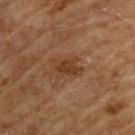Imaged with cross-polarized lighting.
The lesion is located on the back.
A 15 mm crop from a total-body photograph taken for skin-cancer surveillance.
The patient is a male aged around 65.
Automated image analysis of the tile measured a mean CIELAB color near L≈33 a*≈19 b*≈29, a lesion–skin lightness drop of about 7, and a normalized lesion–skin contrast near 7.5. The analysis additionally found an automated nevus-likeness rating near 40 out of 100 and lesion-presence confidence of about 100/100.